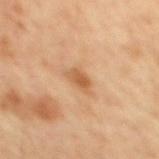biopsy_status: not biopsied; imaged during a skin examination
image:
  source: total-body photography crop
  field_of_view_mm: 15
patient:
  sex: male
  age_approx: 60
automated_metrics:
  area_mm2_approx: 4.0
  shape_asymmetry: 0.3
  border_irregularity_0_10: 3.0
  color_variation_0_10: 3.0
  peripheral_color_asymmetry: 1.0
  nevus_likeness_0_100: 20
lighting: cross-polarized
site: back
lesion_size:
  long_diameter_mm_approx: 2.5